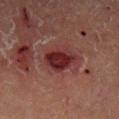biopsy status=catalogued during a skin exam; not biopsied
size=about 4.5 mm
patient=male, aged 53–57
location=the left lower leg
image=~15 mm tile from a whole-body skin photo
tile lighting=cross-polarized